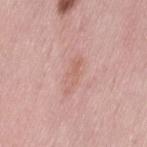Assessment:
The lesion was photographed on a routine skin check and not biopsied; there is no pathology result.
Context:
Cropped from a whole-body photographic skin survey; the tile spans about 15 mm. Located on the leg. The tile uses white-light illumination. The subject is a female aged 48–52.